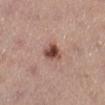{"patient": {"sex": "female", "age_approx": 65}, "site": "left lower leg", "lighting": "white-light", "lesion_size": {"long_diameter_mm_approx": 3.0}, "image": {"source": "total-body photography crop", "field_of_view_mm": 15}, "automated_metrics": {"cielab_L": 47, "cielab_a": 22, "cielab_b": 25, "vs_skin_darker_L": 16.0, "lesion_detection_confidence_0_100": 100}}